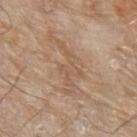The lesion was photographed on a routine skin check and not biopsied; there is no pathology result.
A 15 mm close-up extracted from a 3D total-body photography capture.
Measured at roughly 7.5 mm in maximum diameter.
The lesion is located on the left forearm.
The tile uses white-light illumination.
The patient is a male aged 78 to 82.